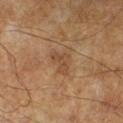* workup · catalogued during a skin exam; not biopsied
* subject · male, aged 63 to 67
* imaging modality · ~15 mm crop, total-body skin-cancer survey
* illumination · cross-polarized illumination